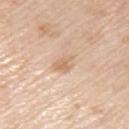- site — the left upper arm
- image — 15 mm crop, total-body photography
- subject — male, aged 78 to 82
- lighting — white-light
- image-analysis metrics — a lesion area of about 4 mm², an eccentricity of roughly 0.75, and a symmetry-axis asymmetry near 0.25; a border-irregularity index near 2.5/10 and peripheral color asymmetry of about 1; an automated nevus-likeness rating near 0 out of 100 and lesion-presence confidence of about 100/100